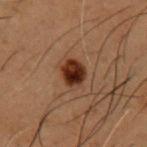Q: How was the tile lit?
A: cross-polarized
Q: What is the imaging modality?
A: ~15 mm tile from a whole-body skin photo
Q: What are the patient's age and sex?
A: male, aged approximately 50
Q: What is the anatomic site?
A: the chest
Q: How large is the lesion?
A: about 3.5 mm